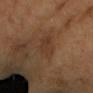Assessment: Imaged during a routine full-body skin examination; the lesion was not biopsied and no histopathology is available. Clinical summary: The lesion is located on the left forearm. Captured under cross-polarized illumination. A region of skin cropped from a whole-body photographic capture, roughly 15 mm wide. The patient is a female aged around 55. About 4 mm across.